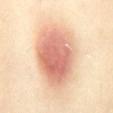Acquisition and patient details:
Imaged with cross-polarized lighting. A 15 mm crop from a total-body photograph taken for skin-cancer surveillance. A female patient, aged around 40. On the mid back.